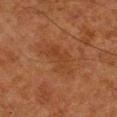Part of a total-body skin-imaging series; this lesion was reviewed on a skin check and was not flagged for biopsy.
This is a cross-polarized tile.
A male patient, approximately 80 years of age.
Automated tile analysis of the lesion measured a lesion area of about 10 mm², an outline eccentricity of about 0.9 (0 = round, 1 = elongated), and a shape-asymmetry score of about 0.35 (0 = symmetric). The software also gave border irregularity of about 4.5 on a 0–10 scale, a color-variation rating of about 2/10, and radial color variation of about 0.5.
Measured at roughly 5.5 mm in maximum diameter.
This image is a 15 mm lesion crop taken from a total-body photograph.
Located on the leg.